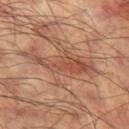Q: Was this lesion biopsied?
A: total-body-photography surveillance lesion; no biopsy
Q: Lesion location?
A: the leg
Q: What kind of image is this?
A: 15 mm crop, total-body photography
Q: Who is the patient?
A: male, aged approximately 60